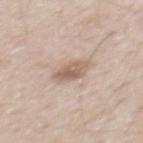{
  "biopsy_status": "not biopsied; imaged during a skin examination",
  "site": "mid back",
  "lighting": "white-light",
  "image": {
    "source": "total-body photography crop",
    "field_of_view_mm": 15
  },
  "patient": {
    "sex": "male",
    "age_approx": 50
  },
  "lesion_size": {
    "long_diameter_mm_approx": 3.5
  },
  "automated_metrics": {
    "cielab_L": 60,
    "cielab_a": 16,
    "cielab_b": 26,
    "vs_skin_contrast_norm": 7.0,
    "nevus_likeness_0_100": 30,
    "lesion_detection_confidence_0_100": 100
  }
}